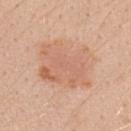Captured during whole-body skin photography for melanoma surveillance; the lesion was not biopsied.
This is a white-light tile.
Longest diameter approximately 6.5 mm.
A region of skin cropped from a whole-body photographic capture, roughly 15 mm wide.
A female patient aged 23–27.
From the arm.